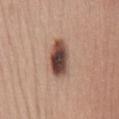Notes:
* biopsy status: imaged on a skin check; not biopsied
* image: ~15 mm crop, total-body skin-cancer survey
* size: ≈5 mm
* subject: female, about 45 years old
* site: the chest
* tile lighting: white-light illumination
* image-analysis metrics: an average lesion color of about L≈46 a*≈19 b*≈24 (CIELAB) and a normalized lesion–skin contrast near 13.5; a border-irregularity index near 2/10, internal color variation of about 8 on a 0–10 scale, and peripheral color asymmetry of about 2; a nevus-likeness score of about 100/100